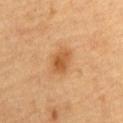Impression: The lesion was photographed on a routine skin check and not biopsied; there is no pathology result. Context: A male patient about 85 years old or older. This is a cross-polarized tile. The lesion is located on the front of the torso. About 3.5 mm across. A 15 mm close-up tile from a total-body photography series done for melanoma screening.